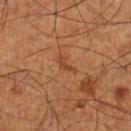Part of a total-body skin-imaging series; this lesion was reviewed on a skin check and was not flagged for biopsy. Located on the left lower leg. A 15 mm close-up extracted from a 3D total-body photography capture. The patient is a male roughly 60 years of age. The total-body-photography lesion software estimated a footprint of about 2.5 mm² and two-axis asymmetry of about 0.6. The software also gave roughly 7 lightness units darker than nearby skin and a normalized lesion–skin contrast near 6. The analysis additionally found lesion-presence confidence of about 100/100. The lesion's longest dimension is about 2.5 mm. Imaged with cross-polarized lighting.